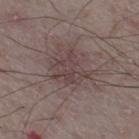Assessment:
Recorded during total-body skin imaging; not selected for excision or biopsy.
Image and clinical context:
A 15 mm crop from a total-body photograph taken for skin-cancer surveillance. The recorded lesion diameter is about 4 mm. On the right thigh. The patient is a male in their mid-70s. The lesion-visualizer software estimated a border-irregularity index near 5/10, a color-variation rating of about 3.5/10, and radial color variation of about 1.5. It also reported a nevus-likeness score of about 0/100 and a detector confidence of about 95 out of 100 that the crop contains a lesion. The tile uses white-light illumination.